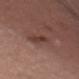The lesion was photographed on a routine skin check and not biopsied; there is no pathology result. The lesion is located on the chest. Approximately 2.5 mm at its widest. A female patient aged 38–42. A close-up tile cropped from a whole-body skin photograph, about 15 mm across. The tile uses white-light illumination.